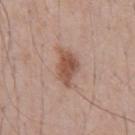biopsy status = no biopsy performed (imaged during a skin exam)
site = the front of the torso
image source = ~15 mm tile from a whole-body skin photo
lesion diameter = ≈4.5 mm
patient = male, aged 68–72